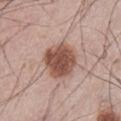follow-up = imaged on a skin check; not biopsied | subject = male, approximately 65 years of age | size = about 5 mm | imaging modality = total-body-photography crop, ~15 mm field of view | lighting = white-light illumination | location = the abdomen.